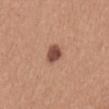Impression:
Recorded during total-body skin imaging; not selected for excision or biopsy.
Background:
Cropped from a whole-body photographic skin survey; the tile spans about 15 mm. A female patient, about 40 years old. The lesion is located on the mid back.